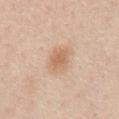This lesion was catalogued during total-body skin photography and was not selected for biopsy. Cropped from a total-body skin-imaging series; the visible field is about 15 mm. A male subject, approximately 60 years of age. On the abdomen. Longest diameter approximately 3.5 mm. The total-body-photography lesion software estimated a border-irregularity rating of about 3/10, a color-variation rating of about 2/10, and radial color variation of about 0.5. And it measured lesion-presence confidence of about 100/100. This is a white-light tile.